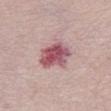Q: Is there a histopathology result?
A: catalogued during a skin exam; not biopsied
Q: Lesion location?
A: the chest
Q: How large is the lesion?
A: ~4.5 mm (longest diameter)
Q: Patient demographics?
A: female, aged around 70
Q: How was this image acquired?
A: ~15 mm tile from a whole-body skin photo
Q: Illumination type?
A: white-light illumination